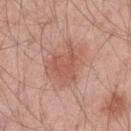The lesion was tiled from a total-body skin photograph and was not biopsied. Automated tile analysis of the lesion measured a border-irregularity rating of about 4/10 and peripheral color asymmetry of about 1. The software also gave a nevus-likeness score of about 90/100 and lesion-presence confidence of about 100/100. Imaged with white-light lighting. Approximately 5.5 mm at its widest. A male subject aged approximately 45. A 15 mm crop from a total-body photograph taken for skin-cancer surveillance. The lesion is located on the arm.